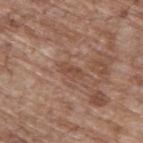{
  "biopsy_status": "not biopsied; imaged during a skin examination",
  "patient": {
    "sex": "male",
    "age_approx": 70
  },
  "lighting": "white-light",
  "lesion_size": {
    "long_diameter_mm_approx": 3.0
  },
  "site": "upper back",
  "image": {
    "source": "total-body photography crop",
    "field_of_view_mm": 15
  }
}